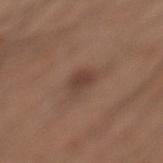The lesion was tiled from a total-body skin photograph and was not biopsied. A male subject, aged 28 to 32. The lesion is on the leg. Approximately 2.5 mm at its widest. Cropped from a total-body skin-imaging series; the visible field is about 15 mm. The tile uses white-light illumination. The lesion-visualizer software estimated a lesion color around L≈41 a*≈18 b*≈24 in CIELAB, about 8 CIELAB-L* units darker than the surrounding skin, and a lesion-to-skin contrast of about 7 (normalized; higher = more distinct).